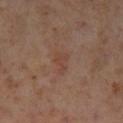A female subject aged 53–57. Approximately 3 mm at its widest. Imaged with cross-polarized lighting. A lesion tile, about 15 mm wide, cut from a 3D total-body photograph. An algorithmic analysis of the crop reported an average lesion color of about L≈43 a*≈20 b*≈27 (CIELAB) and a normalized border contrast of about 5. The analysis additionally found a classifier nevus-likeness of about 0/100 and a lesion-detection confidence of about 100/100. On the left lower leg.